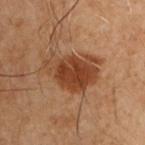{
  "automated_metrics": {
    "area_mm2_approx": 17.0,
    "eccentricity": 0.75,
    "shape_asymmetry": 0.25,
    "cielab_L": 31,
    "cielab_a": 19,
    "cielab_b": 27,
    "vs_skin_contrast_norm": 9.0,
    "border_irregularity_0_10": 3.5,
    "color_variation_0_10": 3.5,
    "nevus_likeness_0_100": 90,
    "lesion_detection_confidence_0_100": 100
  },
  "lesion_size": {
    "long_diameter_mm_approx": 6.0
  },
  "lighting": "cross-polarized",
  "patient": {
    "sex": "male",
    "age_approx": 50
  },
  "image": {
    "source": "total-body photography crop",
    "field_of_view_mm": 15
  },
  "site": "right upper arm"
}